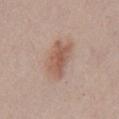A close-up tile cropped from a whole-body skin photograph, about 15 mm across. The lesion-visualizer software estimated roughly 10 lightness units darker than nearby skin and a normalized border contrast of about 7. It also reported border irregularity of about 3 on a 0–10 scale, a within-lesion color-variation index near 3.5/10, and peripheral color asymmetry of about 1. The analysis additionally found a lesion-detection confidence of about 100/100. Measured at roughly 4.5 mm in maximum diameter. A male patient aged approximately 40. On the chest. Captured under white-light illumination.